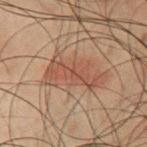Assessment:
Imaged during a routine full-body skin examination; the lesion was not biopsied and no histopathology is available.
Background:
The patient is a male roughly 50 years of age. A 15 mm crop from a total-body photograph taken for skin-cancer surveillance. Located on the chest. Measured at roughly 6.5 mm in maximum diameter. The tile uses cross-polarized illumination.